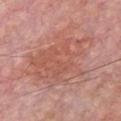Notes:
– diameter · about 10.5 mm
– image · total-body-photography crop, ~15 mm field of view
– illumination · white-light
– site · the chest
– subject · male, about 65 years old